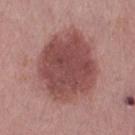Assessment:
No biopsy was performed on this lesion — it was imaged during a full skin examination and was not determined to be concerning.
Image and clinical context:
The lesion-visualizer software estimated an outline eccentricity of about 0.55 (0 = round, 1 = elongated) and a symmetry-axis asymmetry near 0.1. The software also gave a mean CIELAB color near L≈48 a*≈24 b*≈22, a lesion–skin lightness drop of about 13, and a normalized lesion–skin contrast near 9. The analysis additionally found border irregularity of about 1.5 on a 0–10 scale and a within-lesion color-variation index near 3.5/10. And it measured an automated nevus-likeness rating near 75 out of 100 and a detector confidence of about 100 out of 100 that the crop contains a lesion. A female patient, aged 63 to 67. The recorded lesion diameter is about 8 mm. On the leg. The tile uses white-light illumination. A 15 mm close-up tile from a total-body photography series done for melanoma screening.